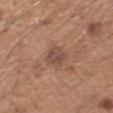Q: Was this lesion biopsied?
A: catalogued during a skin exam; not biopsied
Q: Where on the body is the lesion?
A: the left upper arm
Q: Patient demographics?
A: male, aged 48–52
Q: What kind of image is this?
A: 15 mm crop, total-body photography